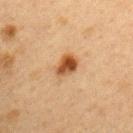A 15 mm crop from a total-body photograph taken for skin-cancer surveillance.
A female subject approximately 40 years of age.
The lesion is located on the left upper arm.
Measured at roughly 3.5 mm in maximum diameter.